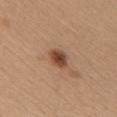The lesion was photographed on a routine skin check and not biopsied; there is no pathology result.
Captured under white-light illumination.
A 15 mm close-up tile from a total-body photography series done for melanoma screening.
A female patient, in their mid-40s.
From the chest.
Approximately 3 mm at its widest.
Automated image analysis of the tile measured an area of roughly 5 mm², an outline eccentricity of about 0.7 (0 = round, 1 = elongated), and two-axis asymmetry of about 0.2. The analysis additionally found a border-irregularity rating of about 2/10, a color-variation rating of about 5/10, and radial color variation of about 1.5. It also reported a classifier nevus-likeness of about 95/100 and a lesion-detection confidence of about 100/100.